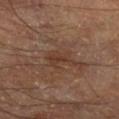| key | value |
|---|---|
| workup | imaged on a skin check; not biopsied |
| lesion size | ~4 mm (longest diameter) |
| patient | male, aged 68–72 |
| automated lesion analysis | an area of roughly 6 mm²; an average lesion color of about L≈36 a*≈18 b*≈26 (CIELAB) and a normalized lesion–skin contrast near 6 |
| anatomic site | the right lower leg |
| acquisition | ~15 mm crop, total-body skin-cancer survey |